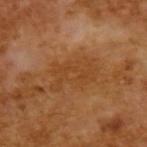Clinical impression: Part of a total-body skin-imaging series; this lesion was reviewed on a skin check and was not flagged for biopsy. Image and clinical context: A close-up tile cropped from a whole-body skin photograph, about 15 mm across. A male subject, roughly 65 years of age. Captured under cross-polarized illumination. The recorded lesion diameter is about 5.5 mm.